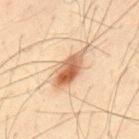<tbp_lesion>
<biopsy_status>not biopsied; imaged during a skin examination</biopsy_status>
<lesion_size>
  <long_diameter_mm_approx>6.0</long_diameter_mm_approx>
</lesion_size>
<image>
  <source>total-body photography crop</source>
  <field_of_view_mm>15</field_of_view_mm>
</image>
<lighting>cross-polarized</lighting>
<patient>
  <sex>male</sex>
  <age_approx>50</age_approx>
</patient>
<automated_metrics>
  <area_mm2_approx>11.0</area_mm2_approx>
  <eccentricity>0.9</eccentricity>
  <shape_asymmetry>0.25</shape_asymmetry>
  <cielab_L>58</cielab_L>
  <cielab_a>19</cielab_a>
  <cielab_b>33</cielab_b>
  <vs_skin_darker_L>14.0</vs_skin_darker_L>
  <vs_skin_contrast_norm>9.0</vs_skin_contrast_norm>
  <border_irregularity_0_10>4.0</border_irregularity_0_10>
  <color_variation_0_10>8.0</color_variation_0_10>
  <peripheral_color_asymmetry>2.5</peripheral_color_asymmetry>
  <nevus_likeness_0_100>95</nevus_likeness_0_100>
</automated_metrics>
<site>upper back</site>
</tbp_lesion>